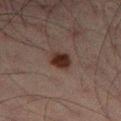Assessment:
Recorded during total-body skin imaging; not selected for excision or biopsy.
Context:
A lesion tile, about 15 mm wide, cut from a 3D total-body photograph. Captured under cross-polarized illumination. On the left thigh. Automated image analysis of the tile measured border irregularity of about 1.5 on a 0–10 scale and internal color variation of about 2.5 on a 0–10 scale. The analysis additionally found a classifier nevus-likeness of about 95/100. The lesion's longest dimension is about 3 mm. The subject is a male aged around 50.